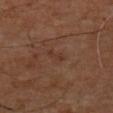This lesion was catalogued during total-body skin photography and was not selected for biopsy.
A region of skin cropped from a whole-body photographic capture, roughly 15 mm wide.
Automated tile analysis of the lesion measured a lesion area of about 2 mm², a shape eccentricity near 0.95, and a shape-asymmetry score of about 0.35 (0 = symmetric). The software also gave an average lesion color of about L≈30 a*≈18 b*≈24 (CIELAB), about 4 CIELAB-L* units darker than the surrounding skin, and a normalized lesion–skin contrast near 5. The software also gave border irregularity of about 5 on a 0–10 scale, a color-variation rating of about 0/10, and peripheral color asymmetry of about 0.
On the front of the torso.
A male subject roughly 60 years of age.
Captured under cross-polarized illumination.